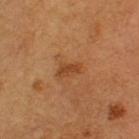Case summary:
- workup · catalogued during a skin exam; not biopsied
- lesion size · ≈2.5 mm
- imaging modality · total-body-photography crop, ~15 mm field of view
- body site · the head or neck
- patient · male, aged approximately 55